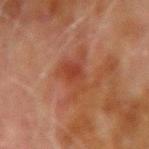anatomic site: the right upper arm
illumination: cross-polarized illumination
patient: male, aged around 70
imaging modality: ~15 mm crop, total-body skin-cancer survey
diameter: ≈4.5 mm